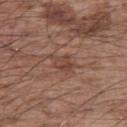Case summary:
– biopsy status · catalogued during a skin exam; not biopsied
– subject · male, aged 63–67
– size · ≈3.5 mm
– anatomic site · the arm
– automated metrics · a lesion area of about 5 mm², an eccentricity of roughly 0.75, and a shape-asymmetry score of about 0.35 (0 = symmetric)
– lighting · white-light
– acquisition · total-body-photography crop, ~15 mm field of view